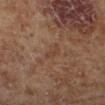<tbp_lesion>
  <biopsy_status>not biopsied; imaged during a skin examination</biopsy_status>
  <image>
    <source>total-body photography crop</source>
    <field_of_view_mm>15</field_of_view_mm>
  </image>
  <lighting>cross-polarized</lighting>
  <site>left lower leg</site>
  <patient>
    <sex>male</sex>
    <age_approx>70</age_approx>
  </patient>
  <lesion_size>
    <long_diameter_mm_approx>3.0</long_diameter_mm_approx>
  </lesion_size>
</tbp_lesion>